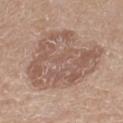Recorded during total-body skin imaging; not selected for excision or biopsy.
This is a white-light tile.
This image is a 15 mm lesion crop taken from a total-body photograph.
From the right thigh.
The patient is a male approximately 65 years of age.
Automated image analysis of the tile measured a lesion color around L≈55 a*≈17 b*≈26 in CIELAB, a lesion–skin lightness drop of about 9, and a normalized lesion–skin contrast near 6. The analysis additionally found a border-irregularity index near 4.5/10, a within-lesion color-variation index near 5/10, and radial color variation of about 1.5. The software also gave an automated nevus-likeness rating near 10 out of 100 and a lesion-detection confidence of about 100/100.
Approximately 8.5 mm at its widest.